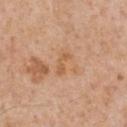This lesion was catalogued during total-body skin photography and was not selected for biopsy. A lesion tile, about 15 mm wide, cut from a 3D total-body photograph. Automated tile analysis of the lesion measured a footprint of about 3 mm² and a shape-asymmetry score of about 0.35 (0 = symmetric). The analysis additionally found roughly 7 lightness units darker than nearby skin and a normalized border contrast of about 6. Longest diameter approximately 3 mm. Imaged with white-light lighting. A male subject approximately 60 years of age. The lesion is located on the front of the torso.